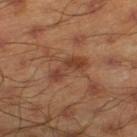biopsy_status: not biopsied; imaged during a skin examination
patient:
  sex: male
  age_approx: 45
lighting: cross-polarized
site: right lower leg
image:
  source: total-body photography crop
  field_of_view_mm: 15
lesion_size:
  long_diameter_mm_approx: 4.5